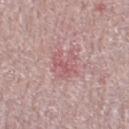No biopsy was performed on this lesion — it was imaged during a full skin examination and was not determined to be concerning. A close-up tile cropped from a whole-body skin photograph, about 15 mm across. From the right thigh. The patient is a male aged 83 or older.